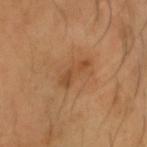Assessment:
Part of a total-body skin-imaging series; this lesion was reviewed on a skin check and was not flagged for biopsy.
Acquisition and patient details:
The total-body-photography lesion software estimated a border-irregularity index near 5/10, internal color variation of about 0.5 on a 0–10 scale, and radial color variation of about 0. The software also gave a nevus-likeness score of about 5/100. From the head or neck. Cropped from a total-body skin-imaging series; the visible field is about 15 mm. A male subject, aged approximately 45. Approximately 4 mm at its widest.